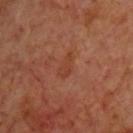This lesion was catalogued during total-body skin photography and was not selected for biopsy.
The lesion's longest dimension is about 3 mm.
A roughly 15 mm field-of-view crop from a total-body skin photograph.
Automated tile analysis of the lesion measured a shape eccentricity near 0.85 and two-axis asymmetry of about 0.3. The analysis additionally found a mean CIELAB color near L≈39 a*≈25 b*≈31 and a normalized lesion–skin contrast near 5. It also reported an automated nevus-likeness rating near 0 out of 100 and a detector confidence of about 100 out of 100 that the crop contains a lesion.
The subject is a male about 70 years old.
This is a cross-polarized tile.
From the front of the torso.